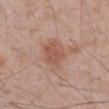Captured during whole-body skin photography for melanoma surveillance; the lesion was not biopsied. About 3.5 mm across. From the abdomen. A male subject, aged approximately 70. This is a white-light tile. A region of skin cropped from a whole-body photographic capture, roughly 15 mm wide.